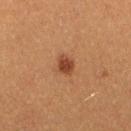Q: Is there a histopathology result?
A: no biopsy performed (imaged during a skin exam)
Q: What lighting was used for the tile?
A: cross-polarized illumination
Q: What are the patient's age and sex?
A: female, aged 38 to 42
Q: Automated lesion metrics?
A: a shape eccentricity near 0.7; an average lesion color of about L≈35 a*≈21 b*≈29 (CIELAB), about 10 CIELAB-L* units darker than the surrounding skin, and a normalized border contrast of about 8.5; radial color variation of about 0.5
Q: What is the imaging modality?
A: ~15 mm tile from a whole-body skin photo
Q: Lesion location?
A: the leg